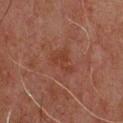No biopsy was performed on this lesion — it was imaged during a full skin examination and was not determined to be concerning.
A region of skin cropped from a whole-body photographic capture, roughly 15 mm wide.
The total-body-photography lesion software estimated a footprint of about 7 mm², an eccentricity of roughly 0.65, and a symmetry-axis asymmetry near 0.55. And it measured a mean CIELAB color near L≈29 a*≈19 b*≈23 and a lesion–skin lightness drop of about 4. And it measured a nevus-likeness score of about 0/100.
The lesion's longest dimension is about 3.5 mm.
A male patient aged around 60.
From the front of the torso.
Imaged with cross-polarized lighting.